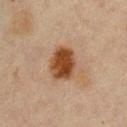Part of a total-body skin-imaging series; this lesion was reviewed on a skin check and was not flagged for biopsy. A female subject aged 43 to 47. The lesion is on the chest. The recorded lesion diameter is about 4 mm. Imaged with cross-polarized lighting. A 15 mm close-up tile from a total-body photography series done for melanoma screening. The total-body-photography lesion software estimated a shape eccentricity near 0.65 and a symmetry-axis asymmetry near 0.1. And it measured a mean CIELAB color near L≈39 a*≈20 b*≈32, a lesion–skin lightness drop of about 14, and a normalized border contrast of about 12. The software also gave a border-irregularity rating of about 1/10, a color-variation rating of about 4/10, and a peripheral color-asymmetry measure near 1.5. The analysis additionally found a nevus-likeness score of about 100/100 and a lesion-detection confidence of about 100/100.